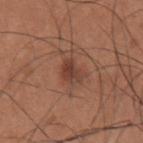Imaged during a routine full-body skin examination; the lesion was not biopsied and no histopathology is available.
From the arm.
A male patient aged 33 to 37.
Cropped from a total-body skin-imaging series; the visible field is about 15 mm.